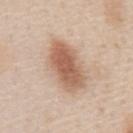biopsy status: catalogued during a skin exam; not biopsied | subject: male, aged around 60 | imaging modality: 15 mm crop, total-body photography | body site: the abdomen.